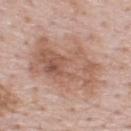biopsy status: imaged on a skin check; not biopsied
illumination: white-light
location: the upper back
subject: male, roughly 50 years of age
lesion diameter: ~9 mm (longest diameter)
image: 15 mm crop, total-body photography